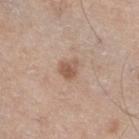workup: no biopsy performed (imaged during a skin exam)
image source: 15 mm crop, total-body photography
lighting: white-light
subject: male, aged approximately 60
site: the right thigh
lesion diameter: ≈2.5 mm
image-analysis metrics: a mean CIELAB color near L≈56 a*≈19 b*≈29 and a normalized lesion–skin contrast near 7; border irregularity of about 3 on a 0–10 scale, internal color variation of about 2.5 on a 0–10 scale, and peripheral color asymmetry of about 1; an automated nevus-likeness rating near 35 out of 100 and lesion-presence confidence of about 100/100